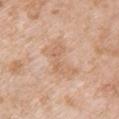Impression:
The lesion was tiled from a total-body skin photograph and was not biopsied.
Clinical summary:
The total-body-photography lesion software estimated an area of roughly 10 mm² and a shape eccentricity near 0.8. The analysis additionally found an average lesion color of about L≈65 a*≈19 b*≈33 (CIELAB), a lesion–skin lightness drop of about 7, and a normalized lesion–skin contrast near 5. A close-up tile cropped from a whole-body skin photograph, about 15 mm across. On the left upper arm. The subject is a female about 70 years old. Captured under white-light illumination.